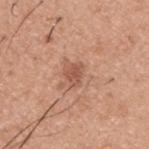Q: Was this lesion biopsied?
A: total-body-photography surveillance lesion; no biopsy
Q: How was the tile lit?
A: white-light illumination
Q: Lesion size?
A: ≈2.5 mm
Q: What are the patient's age and sex?
A: male, aged 38 to 42
Q: What is the anatomic site?
A: the upper back
Q: What is the imaging modality?
A: ~15 mm tile from a whole-body skin photo